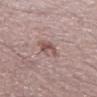  biopsy_status: not biopsied; imaged during a skin examination
  patient:
    sex: male
    age_approx: 50
  image:
    source: total-body photography crop
    field_of_view_mm: 15
  site: left thigh